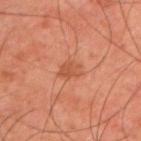No biopsy was performed on this lesion — it was imaged during a full skin examination and was not determined to be concerning. A male subject approximately 45 years of age. The lesion is on the right upper arm. A roughly 15 mm field-of-view crop from a total-body skin photograph.